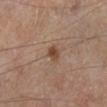Part of a total-body skin-imaging series; this lesion was reviewed on a skin check and was not flagged for biopsy. Cropped from a total-body skin-imaging series; the visible field is about 15 mm. Located on the left lower leg. A male patient roughly 65 years of age. The total-body-photography lesion software estimated a lesion area of about 3 mm², an outline eccentricity of about 0.55 (0 = round, 1 = elongated), and a shape-asymmetry score of about 0.15 (0 = symmetric). The lesion's longest dimension is about 2 mm. The tile uses cross-polarized illumination.